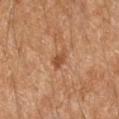A region of skin cropped from a whole-body photographic capture, roughly 15 mm wide. Automated tile analysis of the lesion measured a footprint of about 3.5 mm², a shape eccentricity near 0.9, and two-axis asymmetry of about 0.3. The lesion is located on the right forearm. The subject is a female in their 60s. Imaged with cross-polarized lighting.